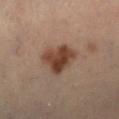Clinical impression: No biopsy was performed on this lesion — it was imaged during a full skin examination and was not determined to be concerning. Image and clinical context: The tile uses cross-polarized illumination. Measured at roughly 5 mm in maximum diameter. On the left lower leg. A region of skin cropped from a whole-body photographic capture, roughly 15 mm wide. A male patient aged approximately 55. The total-body-photography lesion software estimated border irregularity of about 3 on a 0–10 scale and a within-lesion color-variation index near 4.5/10.